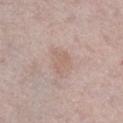A 15 mm close-up tile from a total-body photography series done for melanoma screening. Longest diameter approximately 3.5 mm. The patient is a male aged around 60. Located on the leg.